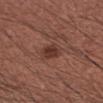notes: total-body-photography surveillance lesion; no biopsy
patient: male, in their 60s
image: total-body-photography crop, ~15 mm field of view
lesion size: about 3 mm
body site: the right forearm
lighting: white-light
automated lesion analysis: a mean CIELAB color near L≈36 a*≈23 b*≈24, about 9 CIELAB-L* units darker than the surrounding skin, and a normalized lesion–skin contrast near 7.5